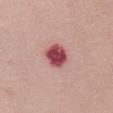follow-up: no biopsy performed (imaged during a skin exam)
subject: female, about 50 years old
location: the chest
image source: ~15 mm crop, total-body skin-cancer survey
lesion diameter: ≈3 mm
tile lighting: white-light